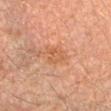biopsy status: imaged on a skin check; not biopsied | subject: male, roughly 35 years of age | image: total-body-photography crop, ~15 mm field of view | body site: the right forearm | tile lighting: cross-polarized.